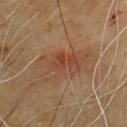Q: Is there a histopathology result?
A: total-body-photography surveillance lesion; no biopsy
Q: What kind of image is this?
A: ~15 mm tile from a whole-body skin photo
Q: What is the anatomic site?
A: the chest
Q: Who is the patient?
A: male, aged approximately 65
Q: How was the tile lit?
A: cross-polarized illumination
Q: Lesion size?
A: about 6 mm